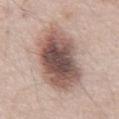Clinical impression: This lesion was catalogued during total-body skin photography and was not selected for biopsy. Background: From the front of the torso. The subject is a male aged around 55. Measured at roughly 11 mm in maximum diameter. A close-up tile cropped from a whole-body skin photograph, about 15 mm across. This is a white-light tile.